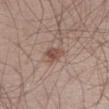<record>
<lesion_size>
  <long_diameter_mm_approx>2.5</long_diameter_mm_approx>
</lesion_size>
<site>right lower leg</site>
<image>
  <source>total-body photography crop</source>
  <field_of_view_mm>15</field_of_view_mm>
</image>
<patient>
  <sex>male</sex>
  <age_approx>35</age_approx>
</patient>
<lighting>white-light</lighting>
<automated_metrics>
  <area_mm2_approx>4.0</area_mm2_approx>
  <eccentricity>0.65</eccentricity>
  <shape_asymmetry>0.35</shape_asymmetry>
</automated_metrics>
</record>